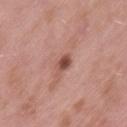No biopsy was performed on this lesion — it was imaged during a full skin examination and was not determined to be concerning. The tile uses white-light illumination. Cropped from a total-body skin-imaging series; the visible field is about 15 mm. A male patient aged approximately 55. The lesion is on the lower back. Automated image analysis of the tile measured about 13 CIELAB-L* units darker than the surrounding skin and a normalized border contrast of about 8.5.